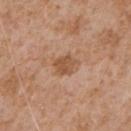workup: total-body-photography surveillance lesion; no biopsy | tile lighting: white-light | body site: the chest | patient: male, aged 63 to 67 | diameter: ~3 mm (longest diameter) | imaging modality: 15 mm crop, total-body photography.